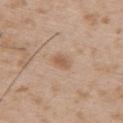Recorded during total-body skin imaging; not selected for excision or biopsy.
The lesion is on the upper back.
A male patient, roughly 55 years of age.
The tile uses white-light illumination.
Cropped from a total-body skin-imaging series; the visible field is about 15 mm.
Measured at roughly 2.5 mm in maximum diameter.